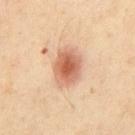Imaged during a routine full-body skin examination; the lesion was not biopsied and no histopathology is available.
Longest diameter approximately 4 mm.
From the abdomen.
The total-body-photography lesion software estimated a nevus-likeness score of about 100/100 and a detector confidence of about 100 out of 100 that the crop contains a lesion.
A male subject, approximately 55 years of age.
This is a cross-polarized tile.
A roughly 15 mm field-of-view crop from a total-body skin photograph.